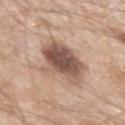Q: Is there a histopathology result?
A: no biopsy performed (imaged during a skin exam)
Q: What is the imaging modality?
A: ~15 mm crop, total-body skin-cancer survey
Q: What lighting was used for the tile?
A: white-light illumination
Q: What are the patient's age and sex?
A: male, approximately 55 years of age
Q: Lesion location?
A: the left upper arm
Q: What did automated image analysis measure?
A: a footprint of about 18 mm², an eccentricity of roughly 0.65, and two-axis asymmetry of about 0.25; internal color variation of about 6 on a 0–10 scale and a peripheral color-asymmetry measure near 1.5
Q: What is the lesion's diameter?
A: ~5.5 mm (longest diameter)